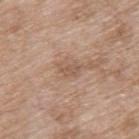Notes:
• notes: imaged on a skin check; not biopsied
• subject: female, roughly 75 years of age
• lesion diameter: ~2.5 mm (longest diameter)
• acquisition: ~15 mm tile from a whole-body skin photo
• body site: the upper back
• illumination: white-light illumination